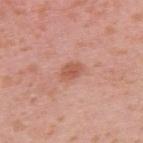Q: Was this lesion biopsied?
A: catalogued during a skin exam; not biopsied
Q: What is the imaging modality?
A: 15 mm crop, total-body photography
Q: What is the anatomic site?
A: the upper back
Q: What are the patient's age and sex?
A: female, approximately 40 years of age
Q: What lighting was used for the tile?
A: white-light illumination
Q: Lesion size?
A: about 3 mm
Q: Automated lesion metrics?
A: a border-irregularity index near 2.5/10 and a within-lesion color-variation index near 1.5/10; a nevus-likeness score of about 25/100 and a lesion-detection confidence of about 100/100